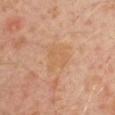notes: no biopsy performed (imaged during a skin exam); site: the left arm; lighting: cross-polarized; lesion diameter: ~3.5 mm (longest diameter); subject: male, aged 28 to 32; image source: total-body-photography crop, ~15 mm field of view.